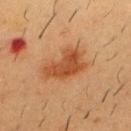From the chest.
About 5 mm across.
A region of skin cropped from a whole-body photographic capture, roughly 15 mm wide.
The subject is a male in their mid-50s.